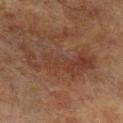<record>
<biopsy_status>not biopsied; imaged during a skin examination</biopsy_status>
<site>leg</site>
<patient>
  <sex>male</sex>
  <age_approx>70</age_approx>
</patient>
<automated_metrics>
  <area_mm2_approx>19.0</area_mm2_approx>
  <eccentricity>0.9</eccentricity>
  <shape_asymmetry>0.45</shape_asymmetry>
  <cielab_L>31</cielab_L>
  <cielab_a>18</cielab_a>
  <cielab_b>23</cielab_b>
  <vs_skin_darker_L>5.0</vs_skin_darker_L>
  <vs_skin_contrast_norm>5.5</vs_skin_contrast_norm>
  <nevus_likeness_0_100>0</nevus_likeness_0_100>
  <lesion_detection_confidence_0_100>100</lesion_detection_confidence_0_100>
</automated_metrics>
<lighting>cross-polarized</lighting>
<image>
  <source>total-body photography crop</source>
  <field_of_view_mm>15</field_of_view_mm>
</image>
</record>